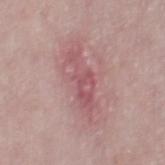No biopsy was performed on this lesion — it was imaged during a full skin examination and was not determined to be concerning. This image is a 15 mm lesion crop taken from a total-body photograph. Longest diameter approximately 6.5 mm. Located on the right thigh. A female subject about 50 years old. An algorithmic analysis of the crop reported a mean CIELAB color near L≈54 a*≈26 b*≈18 and a normalized border contrast of about 6.5. And it measured a border-irregularity rating of about 6.5/10, a color-variation rating of about 2.5/10, and peripheral color asymmetry of about 1.